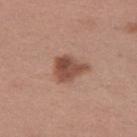  biopsy_status: not biopsied; imaged during a skin examination
  site: left thigh
  patient:
    sex: female
    age_approx: 30
  image:
    source: total-body photography crop
    field_of_view_mm: 15